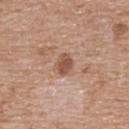follow-up: catalogued during a skin exam; not biopsied
imaging modality: 15 mm crop, total-body photography
patient: female, in their 70s
illumination: white-light illumination
size: ~2.5 mm (longest diameter)
automated metrics: a within-lesion color-variation index near 1.5/10 and peripheral color asymmetry of about 0.5; an automated nevus-likeness rating near 90 out of 100 and a lesion-detection confidence of about 100/100
anatomic site: the upper back